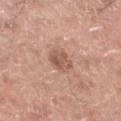  lesion_size:
    long_diameter_mm_approx: 3.0
  image:
    source: total-body photography crop
    field_of_view_mm: 15
  site: left lower leg
  lighting: white-light
  patient:
    sex: male
    age_approx: 55